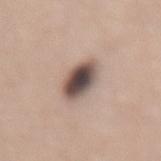– notes · no biopsy performed (imaged during a skin exam)
– automated lesion analysis · an automated nevus-likeness rating near 70 out of 100 and a detector confidence of about 100 out of 100 that the crop contains a lesion
– imaging modality · 15 mm crop, total-body photography
– size · ≈4 mm
– tile lighting · white-light
– patient · female, approximately 55 years of age
– anatomic site · the lower back